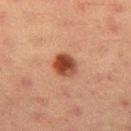follow-up=no biopsy performed (imaged during a skin exam)
body site=the left thigh
imaging modality=total-body-photography crop, ~15 mm field of view
lighting=cross-polarized illumination
TBP lesion metrics=an eccentricity of roughly 0.5 and two-axis asymmetry of about 0.15; an average lesion color of about L≈37 a*≈24 b*≈28 (CIELAB); a classifier nevus-likeness of about 100/100
subject=female, in their mid-50s
size=≈3 mm